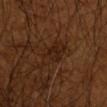workup — no biopsy performed (imaged during a skin exam) | lighting — cross-polarized illumination | subject — male, in their mid- to late 60s | acquisition — ~15 mm crop, total-body skin-cancer survey | lesion size — ≈4.5 mm | anatomic site — the right upper arm.